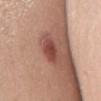• biopsy status: catalogued during a skin exam; not biopsied
• patient: female, in their mid- to late 40s
• imaging modality: ~15 mm tile from a whole-body skin photo
• lighting: white-light illumination
• anatomic site: the chest
• diameter: ~3.5 mm (longest diameter)